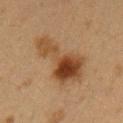  biopsy_status: not biopsied; imaged during a skin examination
  patient:
    sex: female
    age_approx: 40
  site: left upper arm
  image:
    source: total-body photography crop
    field_of_view_mm: 15